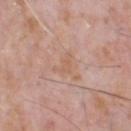Clinical impression:
Recorded during total-body skin imaging; not selected for excision or biopsy.
Background:
From the head or neck. The subject is a male aged approximately 70. The total-body-photography lesion software estimated an area of roughly 3.5 mm², a shape eccentricity near 0.75, and two-axis asymmetry of about 0.55. It also reported a border-irregularity index near 6.5/10, a color-variation rating of about 0.5/10, and peripheral color asymmetry of about 0.5. The software also gave a classifier nevus-likeness of about 0/100 and lesion-presence confidence of about 100/100. A lesion tile, about 15 mm wide, cut from a 3D total-body photograph. The tile uses white-light illumination. The recorded lesion diameter is about 3 mm.